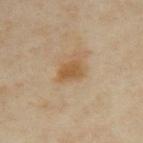| field | value |
|---|---|
| workup | total-body-photography surveillance lesion; no biopsy |
| lighting | cross-polarized illumination |
| size | ≈3 mm |
| site | the left upper arm |
| subject | female, aged 33–37 |
| TBP lesion metrics | a lesion area of about 5 mm² |
| imaging modality | ~15 mm crop, total-body skin-cancer survey |